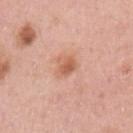notes — catalogued during a skin exam; not biopsied | image — 15 mm crop, total-body photography | subject — female, aged 38–42 | location — the arm.